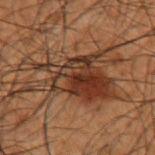This lesion was catalogued during total-body skin photography and was not selected for biopsy.
Located on the left forearm.
A region of skin cropped from a whole-body photographic capture, roughly 15 mm wide.
Captured under cross-polarized illumination.
The lesion's longest dimension is about 8.5 mm.
The subject is a male approximately 50 years of age.